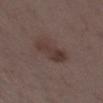<case>
  <biopsy_status>not biopsied; imaged during a skin examination</biopsy_status>
  <lighting>white-light</lighting>
  <site>right thigh</site>
  <automated_metrics>
    <nevus_likeness_0_100>10</nevus_likeness_0_100>
    <lesion_detection_confidence_0_100>100</lesion_detection_confidence_0_100>
  </automated_metrics>
  <lesion_size>
    <long_diameter_mm_approx>5.0</long_diameter_mm_approx>
  </lesion_size>
  <patient>
    <sex>female</sex>
    <age_approx>35</age_approx>
  </patient>
  <image>
    <source>total-body photography crop</source>
    <field_of_view_mm>15</field_of_view_mm>
  </image>
</case>